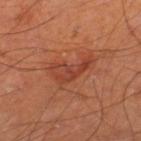<lesion>
<biopsy_status>not biopsied; imaged during a skin examination</biopsy_status>
<patient>
  <sex>male</sex>
  <age_approx>65</age_approx>
</patient>
<image>
  <source>total-body photography crop</source>
  <field_of_view_mm>15</field_of_view_mm>
</image>
<lighting>cross-polarized</lighting>
<site>left thigh</site>
<lesion_size>
  <long_diameter_mm_approx>5.0</long_diameter_mm_approx>
</lesion_size>
</lesion>